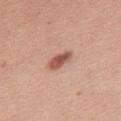Cropped from a whole-body photographic skin survey; the tile spans about 15 mm.
From the upper back.
A female subject aged around 35.